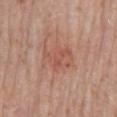Impression: Recorded during total-body skin imaging; not selected for excision or biopsy. Context: A male subject, in their mid- to late 70s. Cropped from a whole-body photographic skin survey; the tile spans about 15 mm. Imaged with white-light lighting. Measured at roughly 4 mm in maximum diameter. The lesion-visualizer software estimated a lesion area of about 11 mm² and a shape eccentricity near 0.75. The analysis additionally found roughly 7 lightness units darker than nearby skin and a normalized lesion–skin contrast near 5. The analysis additionally found a border-irregularity rating of about 3/10. The lesion is located on the mid back.